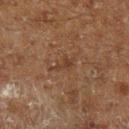Captured during whole-body skin photography for melanoma surveillance; the lesion was not biopsied. The lesion is located on the left lower leg. This is a cross-polarized tile. A region of skin cropped from a whole-body photographic capture, roughly 15 mm wide. A male patient, approximately 60 years of age. Longest diameter approximately 3 mm. Automated image analysis of the tile measured a shape eccentricity near 0.85 and a symmetry-axis asymmetry near 0.4. And it measured a lesion-detection confidence of about 100/100.